A female subject about 70 years old. From the right forearm. A 15 mm close-up tile from a total-body photography series done for melanoma screening.
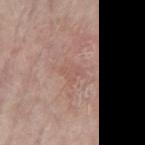The lesion was biopsied, and histopathology showed an actinic keratosis, classified as a lesion of indeterminate malignant potential.